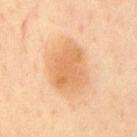Captured during whole-body skin photography for melanoma surveillance; the lesion was not biopsied. A male subject in their mid-50s. A lesion tile, about 15 mm wide, cut from a 3D total-body photograph. Approximately 6 mm at its widest. From the chest. Imaged with cross-polarized lighting. An algorithmic analysis of the crop reported an area of roughly 20 mm², an outline eccentricity of about 0.65 (0 = round, 1 = elongated), and a symmetry-axis asymmetry near 0.2. The analysis additionally found a border-irregularity rating of about 2/10, a within-lesion color-variation index near 3/10, and a peripheral color-asymmetry measure near 1.